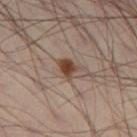No biopsy was performed on this lesion — it was imaged during a full skin examination and was not determined to be concerning. Longest diameter approximately 3 mm. A 15 mm close-up tile from a total-body photography series done for melanoma screening. From the leg. Captured under cross-polarized illumination. The patient is a male aged 38–42.